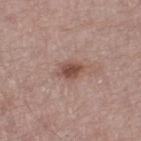notes: imaged on a skin check; not biopsied | subject: male, aged 53 to 57 | lighting: white-light | location: the left thigh | lesion size: ~3 mm (longest diameter) | automated metrics: a lesion area of about 4.5 mm², an outline eccentricity of about 0.7 (0 = round, 1 = elongated), and a shape-asymmetry score of about 0.35 (0 = symmetric); an average lesion color of about L≈49 a*≈20 b*≈24 (CIELAB), about 12 CIELAB-L* units darker than the surrounding skin, and a lesion-to-skin contrast of about 8.5 (normalized; higher = more distinct); a nevus-likeness score of about 80/100 and a detector confidence of about 100 out of 100 that the crop contains a lesion | image: 15 mm crop, total-body photography.